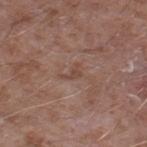Clinical impression: Captured during whole-body skin photography for melanoma surveillance; the lesion was not biopsied. Image and clinical context: A male patient roughly 60 years of age. A close-up tile cropped from a whole-body skin photograph, about 15 mm across. On the left thigh.